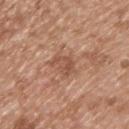Recorded during total-body skin imaging; not selected for excision or biopsy. Located on the upper back. A 15 mm crop from a total-body photograph taken for skin-cancer surveillance. The subject is a male aged 63–67.